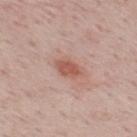Clinical impression: The lesion was tiled from a total-body skin photograph and was not biopsied. Acquisition and patient details: The lesion's longest dimension is about 3.5 mm. A male patient approximately 50 years of age. A lesion tile, about 15 mm wide, cut from a 3D total-body photograph. Located on the mid back.